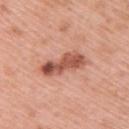Q: Is there a histopathology result?
A: imaged on a skin check; not biopsied
Q: What lighting was used for the tile?
A: white-light
Q: What is the anatomic site?
A: the upper back
Q: How was this image acquired?
A: total-body-photography crop, ~15 mm field of view
Q: How large is the lesion?
A: ≈6 mm
Q: Who is the patient?
A: male, aged around 60